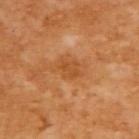follow-up — total-body-photography surveillance lesion; no biopsy | subject — female, aged around 55 | size — ≈3 mm | site — the upper back | tile lighting — cross-polarized illumination | acquisition — ~15 mm tile from a whole-body skin photo.